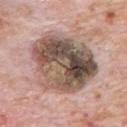<tbp_lesion>
<biopsy_status>not biopsied; imaged during a skin examination</biopsy_status>
<patient>
  <sex>male</sex>
  <age_approx>80</age_approx>
</patient>
<lighting>white-light</lighting>
<automated_metrics>
  <area_mm2_approx>42.0</area_mm2_approx>
  <eccentricity>0.6</eccentricity>
  <shape_asymmetry>0.15</shape_asymmetry>
  <cielab_L>51</cielab_L>
  <cielab_a>15</cielab_a>
  <cielab_b>24</cielab_b>
  <vs_skin_darker_L>17.0</vs_skin_darker_L>
  <vs_skin_contrast_norm>11.0</vs_skin_contrast_norm>
  <nevus_likeness_0_100>20</nevus_likeness_0_100>
  <lesion_detection_confidence_0_100>100</lesion_detection_confidence_0_100>
</automated_metrics>
<lesion_size>
  <long_diameter_mm_approx>8.5</long_diameter_mm_approx>
</lesion_size>
<site>chest</site>
<image>
  <source>total-body photography crop</source>
  <field_of_view_mm>15</field_of_view_mm>
</image>
</tbp_lesion>